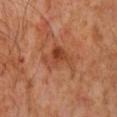Captured during whole-body skin photography for melanoma surveillance; the lesion was not biopsied. The lesion is on the mid back. A male patient, aged approximately 60. The total-body-photography lesion software estimated a lesion area of about 8.5 mm² and a shape eccentricity near 0.75. And it measured a border-irregularity index near 5/10 and a color-variation rating of about 6/10. The tile uses cross-polarized illumination. A close-up tile cropped from a whole-body skin photograph, about 15 mm across.